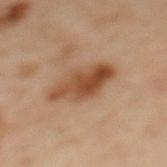notes = imaged on a skin check; not biopsied | imaging modality = ~15 mm tile from a whole-body skin photo | diameter = ~6 mm (longest diameter) | body site = the upper back | patient = female, aged 58 to 62 | tile lighting = cross-polarized.